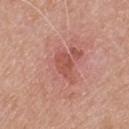Q: Is there a histopathology result?
A: total-body-photography surveillance lesion; no biopsy
Q: What is the imaging modality?
A: ~15 mm crop, total-body skin-cancer survey
Q: What did automated image analysis measure?
A: a shape-asymmetry score of about 0.25 (0 = symmetric)
Q: Patient demographics?
A: male, roughly 65 years of age
Q: How large is the lesion?
A: about 2.5 mm
Q: Illumination type?
A: white-light illumination
Q: Lesion location?
A: the upper back